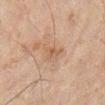anatomic site: the right lower leg
automated metrics: an area of roughly 3.5 mm², an eccentricity of roughly 0.85, and a symmetry-axis asymmetry near 0.35; an average lesion color of about L≈46 a*≈15 b*≈27 (CIELAB), about 6 CIELAB-L* units darker than the surrounding skin, and a normalized lesion–skin contrast near 5.5; border irregularity of about 3.5 on a 0–10 scale, a within-lesion color-variation index near 3/10, and a peripheral color-asymmetry measure near 1; an automated nevus-likeness rating near 0 out of 100
imaging modality: total-body-photography crop, ~15 mm field of view
lighting: cross-polarized illumination
patient: male, about 45 years old